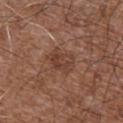Imaged during a routine full-body skin examination; the lesion was not biopsied and no histopathology is available.
Located on the front of the torso.
A 15 mm close-up tile from a total-body photography series done for melanoma screening.
A male subject, aged 73–77.